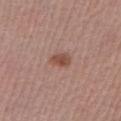{
  "biopsy_status": "not biopsied; imaged during a skin examination",
  "image": {
    "source": "total-body photography crop",
    "field_of_view_mm": 15
  },
  "lighting": "white-light",
  "patient": {
    "sex": "male",
    "age_approx": 55
  },
  "site": "left lower leg",
  "lesion_size": {
    "long_diameter_mm_approx": 2.5
  }
}